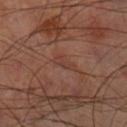Notes:
– location — the right thigh
– diameter — ~2.5 mm (longest diameter)
– image — ~15 mm crop, total-body skin-cancer survey
– lighting — cross-polarized illumination